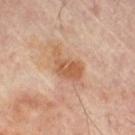Captured during whole-body skin photography for melanoma surveillance; the lesion was not biopsied. The patient is a male roughly 70 years of age. A region of skin cropped from a whole-body photographic capture, roughly 15 mm wide. Located on the right thigh. About 4.5 mm across. Imaged with cross-polarized lighting. Automated image analysis of the tile measured a mean CIELAB color near L≈56 a*≈22 b*≈34, roughly 10 lightness units darker than nearby skin, and a lesion-to-skin contrast of about 7.5 (normalized; higher = more distinct).